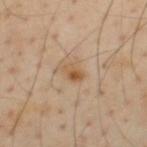The lesion was tiled from a total-body skin photograph and was not biopsied. A male patient aged 53 to 57. Located on the upper back. A roughly 15 mm field-of-view crop from a total-body skin photograph. Approximately 2.5 mm at its widest. Captured under cross-polarized illumination.